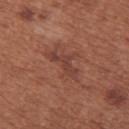Captured during whole-body skin photography for melanoma surveillance; the lesion was not biopsied. A female patient aged 63–67. Imaged with white-light lighting. The lesion is on the upper back. A 15 mm close-up tile from a total-body photography series done for melanoma screening. The total-body-photography lesion software estimated an average lesion color of about L≈41 a*≈24 b*≈26 (CIELAB) and a normalized lesion–skin contrast near 6. The analysis additionally found border irregularity of about 7 on a 0–10 scale, a color-variation rating of about 1.5/10, and peripheral color asymmetry of about 0.